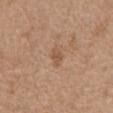This lesion was catalogued during total-body skin photography and was not selected for biopsy. Imaged with white-light lighting. A female patient in their mid- to late 70s. Measured at roughly 2.5 mm in maximum diameter. A roughly 15 mm field-of-view crop from a total-body skin photograph. On the mid back.